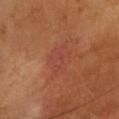Captured during whole-body skin photography for melanoma surveillance; the lesion was not biopsied.
About 4 mm across.
The subject is a female in their mid- to late 50s.
On the head or neck.
A lesion tile, about 15 mm wide, cut from a 3D total-body photograph.
The tile uses cross-polarized illumination.